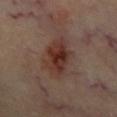Recorded during total-body skin imaging; not selected for excision or biopsy.
On the chest.
Cropped from a whole-body photographic skin survey; the tile spans about 15 mm.
About 6.5 mm across.